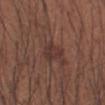A male subject aged 33 to 37. Automated image analysis of the tile measured an eccentricity of roughly 0.6. And it measured a mean CIELAB color near L≈34 a*≈19 b*≈21, about 7 CIELAB-L* units darker than the surrounding skin, and a normalized border contrast of about 6.5. The lesion is located on the right forearm. A 15 mm close-up tile from a total-body photography series done for melanoma screening.